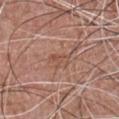This lesion was catalogued during total-body skin photography and was not selected for biopsy. Approximately 2.5 mm at its widest. A male subject aged 53 to 57. From the chest. Imaged with white-light lighting. A 15 mm close-up extracted from a 3D total-body photography capture.